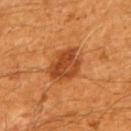Captured during whole-body skin photography for melanoma surveillance; the lesion was not biopsied. Captured under cross-polarized illumination. Approximately 4.5 mm at its widest. The subject is a male approximately 60 years of age. From the mid back. The total-body-photography lesion software estimated a lesion color around L≈43 a*≈28 b*≈39 in CIELAB, a lesion–skin lightness drop of about 11, and a normalized lesion–skin contrast near 8.5. A close-up tile cropped from a whole-body skin photograph, about 15 mm across.